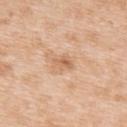<record>
<biopsy_status>not biopsied; imaged during a skin examination</biopsy_status>
<automated_metrics>
  <cielab_L>64</cielab_L>
  <cielab_a>20</cielab_a>
  <cielab_b>35</cielab_b>
  <vs_skin_darker_L>9.0</vs_skin_darker_L>
</automated_metrics>
<site>back</site>
<image>
  <source>total-body photography crop</source>
  <field_of_view_mm>15</field_of_view_mm>
</image>
<patient>
  <sex>female</sex>
  <age_approx>40</age_approx>
</patient>
</record>